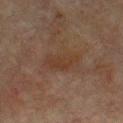Acquisition and patient details: On the upper back. A male patient, aged 63 to 67. A roughly 15 mm field-of-view crop from a total-body skin photograph. This is a cross-polarized tile. The lesion's longest dimension is about 4 mm.